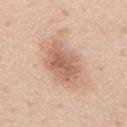{"biopsy_status": "not biopsied; imaged during a skin examination"}